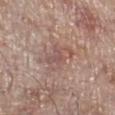workup = total-body-photography surveillance lesion; no biopsy
illumination = white-light
body site = the right lower leg
lesion diameter = about 5 mm
image = ~15 mm crop, total-body skin-cancer survey
subject = male, aged around 70
automated lesion analysis = a mean CIELAB color near L≈54 a*≈19 b*≈23, about 8 CIELAB-L* units darker than the surrounding skin, and a normalized lesion–skin contrast near 5.5; a border-irregularity rating of about 5/10